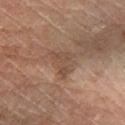No biopsy was performed on this lesion — it was imaged during a full skin examination and was not determined to be concerning.
Captured under cross-polarized illumination.
The lesion is located on the right leg.
A 15 mm close-up extracted from a 3D total-body photography capture.
The recorded lesion diameter is about 3.5 mm.
A male patient, aged approximately 60.
The lesion-visualizer software estimated a within-lesion color-variation index near 2/10 and radial color variation of about 0.5.